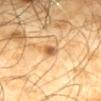| field | value |
|---|---|
| workup | catalogued during a skin exam; not biopsied |
| anatomic site | the mid back |
| size | about 2.5 mm |
| tile lighting | cross-polarized illumination |
| image-analysis metrics | an eccentricity of roughly 0.85 and two-axis asymmetry of about 0.3; a normalized border contrast of about 9; border irregularity of about 2.5 on a 0–10 scale, a within-lesion color-variation index near 3.5/10, and radial color variation of about 1; a classifier nevus-likeness of about 85/100 and a lesion-detection confidence of about 100/100 |
| patient | male, approximately 65 years of age |
| imaging modality | 15 mm crop, total-body photography |